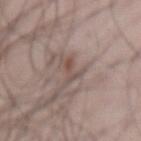Clinical impression:
Captured during whole-body skin photography for melanoma surveillance; the lesion was not biopsied.
Background:
A male patient, aged around 45. Located on the mid back. A 15 mm close-up extracted from a 3D total-body photography capture.